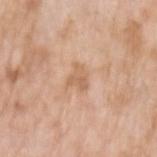workup: no biopsy performed (imaged during a skin exam) | subject: female, aged approximately 75 | anatomic site: the left upper arm | lesion size: ~2.5 mm (longest diameter) | lighting: white-light illumination | imaging modality: ~15 mm tile from a whole-body skin photo | image-analysis metrics: a lesion area of about 4 mm², an outline eccentricity of about 0.6 (0 = round, 1 = elongated), and two-axis asymmetry of about 0.45; an average lesion color of about L≈62 a*≈20 b*≈34 (CIELAB) and a normalized border contrast of about 5.5; a border-irregularity index near 4.5/10, a color-variation rating of about 1.5/10, and peripheral color asymmetry of about 0.5; a nevus-likeness score of about 0/100 and a detector confidence of about 100 out of 100 that the crop contains a lesion.